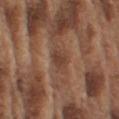Impression: No biopsy was performed on this lesion — it was imaged during a full skin examination and was not determined to be concerning. Clinical summary: A 15 mm crop from a total-body photograph taken for skin-cancer surveillance. On the left upper arm. A male patient, in their mid-70s.